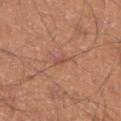Q: Was this lesion biopsied?
A: no biopsy performed (imaged during a skin exam)
Q: What are the patient's age and sex?
A: male, roughly 45 years of age
Q: Lesion location?
A: the left lower leg
Q: How large is the lesion?
A: ≈2.5 mm
Q: What kind of image is this?
A: ~15 mm tile from a whole-body skin photo
Q: What lighting was used for the tile?
A: white-light